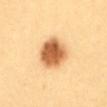- workup: no biopsy performed (imaged during a skin exam)
- patient: female, aged approximately 55
- lesion size: ~4 mm (longest diameter)
- image: total-body-photography crop, ~15 mm field of view
- illumination: cross-polarized illumination
- location: the front of the torso